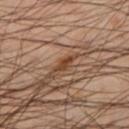Q: What lighting was used for the tile?
A: cross-polarized illumination
Q: Patient demographics?
A: male, aged approximately 50
Q: How was this image acquired?
A: ~15 mm tile from a whole-body skin photo
Q: What is the lesion's diameter?
A: about 2.5 mm
Q: What is the anatomic site?
A: the left lower leg